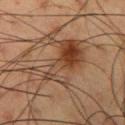The lesion was photographed on a routine skin check and not biopsied; there is no pathology result. An algorithmic analysis of the crop reported a footprint of about 19 mm² and an eccentricity of roughly 0.85. It also reported a lesion color around L≈37 a*≈17 b*≈27 in CIELAB and a lesion–skin lightness drop of about 9. The software also gave a border-irregularity rating of about 9/10 and a color-variation rating of about 9/10. Imaged with cross-polarized lighting. Cropped from a total-body skin-imaging series; the visible field is about 15 mm. A male patient, aged around 55. Measured at roughly 8.5 mm in maximum diameter. From the right upper arm.